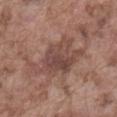{"biopsy_status": "not biopsied; imaged during a skin examination", "patient": {"sex": "male", "age_approx": 75}, "image": {"source": "total-body photography crop", "field_of_view_mm": 15}, "site": "front of the torso"}